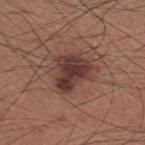biopsy_status: not biopsied; imaged during a skin examination
lighting: white-light
patient:
  sex: male
  age_approx: 35
lesion_size:
  long_diameter_mm_approx: 5.5
image:
  source: total-body photography crop
  field_of_view_mm: 15
site: upper back